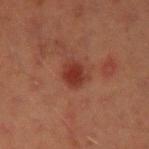<record>
<biopsy_status>not biopsied; imaged during a skin examination</biopsy_status>
<lesion_size>
  <long_diameter_mm_approx>3.0</long_diameter_mm_approx>
</lesion_size>
<lighting>cross-polarized</lighting>
<patient>
  <sex>male</sex>
  <age_approx>45</age_approx>
</patient>
<image>
  <source>total-body photography crop</source>
  <field_of_view_mm>15</field_of_view_mm>
</image>
<site>left upper arm</site>
<automated_metrics>
  <cielab_L>29</cielab_L>
  <cielab_a>24</cielab_a>
  <cielab_b>25</cielab_b>
  <vs_skin_darker_L>8.0</vs_skin_darker_L>
  <vs_skin_contrast_norm>8.0</vs_skin_contrast_norm>
  <border_irregularity_0_10>2.0</border_irregularity_0_10>
  <color_variation_0_10>3.5</color_variation_0_10>
  <peripheral_color_asymmetry>1.0</peripheral_color_asymmetry>
  <nevus_likeness_0_100>90</nevus_likeness_0_100>
  <lesion_detection_confidence_0_100>100</lesion_detection_confidence_0_100>
</automated_metrics>
</record>